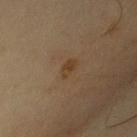Imaged during a routine full-body skin examination; the lesion was not biopsied and no histopathology is available. A 15 mm close-up extracted from a 3D total-body photography capture. Measured at roughly 3 mm in maximum diameter. The patient is a male aged approximately 35. The tile uses cross-polarized illumination. Automated image analysis of the tile measured an outline eccentricity of about 0.9 (0 = round, 1 = elongated) and a symmetry-axis asymmetry near 0.3. The software also gave a lesion color around L≈34 a*≈14 b*≈28 in CIELAB, roughly 6 lightness units darker than nearby skin, and a normalized border contrast of about 7. The software also gave a color-variation rating of about 0.5/10 and a peripheral color-asymmetry measure near 0. And it measured a detector confidence of about 100 out of 100 that the crop contains a lesion. The lesion is located on the chest.